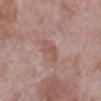Clinical impression:
Captured during whole-body skin photography for melanoma surveillance; the lesion was not biopsied.
Background:
Located on the leg. The subject is a female aged 68 to 72. A close-up tile cropped from a whole-body skin photograph, about 15 mm across.